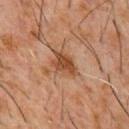No biopsy was performed on this lesion — it was imaged during a full skin examination and was not determined to be concerning.
A male subject, aged around 60.
Approximately 4 mm at its widest.
On the chest.
An algorithmic analysis of the crop reported a lesion area of about 7 mm², a shape eccentricity near 0.8, and a shape-asymmetry score of about 0.35 (0 = symmetric). The software also gave about 10 CIELAB-L* units darker than the surrounding skin and a normalized border contrast of about 8.5. The analysis additionally found internal color variation of about 4 on a 0–10 scale and a peripheral color-asymmetry measure near 1.5.
This is a cross-polarized tile.
A 15 mm crop from a total-body photograph taken for skin-cancer surveillance.